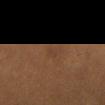Q: Was a biopsy performed?
A: catalogued during a skin exam; not biopsied
Q: How was the tile lit?
A: cross-polarized illumination
Q: Patient demographics?
A: female, aged 38 to 42
Q: How was this image acquired?
A: ~15 mm crop, total-body skin-cancer survey
Q: Where on the body is the lesion?
A: the right thigh